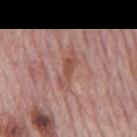A close-up tile cropped from a whole-body skin photograph, about 15 mm across. Located on the mid back. Captured under white-light illumination. A male subject in their mid-70s.